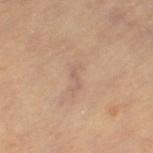<record>
  <image>
    <source>total-body photography crop</source>
    <field_of_view_mm>15</field_of_view_mm>
  </image>
  <patient>
    <sex>female</sex>
    <age_approx>70</age_approx>
  </patient>
  <site>left thigh</site>
</record>